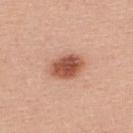Q: Is there a histopathology result?
A: catalogued during a skin exam; not biopsied
Q: Illumination type?
A: white-light illumination
Q: Lesion size?
A: ~4 mm (longest diameter)
Q: How was this image acquired?
A: 15 mm crop, total-body photography
Q: Patient demographics?
A: female, aged 38 to 42
Q: Lesion location?
A: the upper back